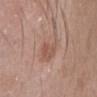Impression:
Captured during whole-body skin photography for melanoma surveillance; the lesion was not biopsied.
Context:
The recorded lesion diameter is about 4.5 mm. This is a white-light tile. Located on the head or neck. A male subject roughly 40 years of age. A roughly 15 mm field-of-view crop from a total-body skin photograph.